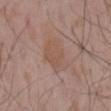Context:
The subject is a male aged 53–57. The tile uses white-light illumination. A close-up tile cropped from a whole-body skin photograph, about 15 mm across. Located on the back. Longest diameter approximately 4.5 mm.